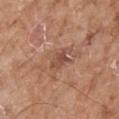The lesion was photographed on a routine skin check and not biopsied; there is no pathology result.
The lesion is on the right forearm.
About 3 mm across.
The patient is a female aged 73 to 77.
The tile uses white-light illumination.
Cropped from a whole-body photographic skin survey; the tile spans about 15 mm.